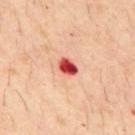Assessment:
No biopsy was performed on this lesion — it was imaged during a full skin examination and was not determined to be concerning.
Clinical summary:
A male subject about 30 years old. A 15 mm crop from a total-body photograph taken for skin-cancer surveillance. On the back. The recorded lesion diameter is about 2.5 mm.